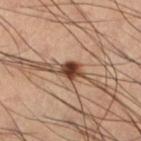biopsy status — imaged on a skin check; not biopsied
location — the left lower leg
lighting — cross-polarized illumination
automated metrics — an eccentricity of roughly 0.45 and two-axis asymmetry of about 0.2; a mean CIELAB color near L≈38 a*≈22 b*≈29, about 21 CIELAB-L* units darker than the surrounding skin, and a normalized lesion–skin contrast near 15.5; a border-irregularity index near 1.5/10, a within-lesion color-variation index near 2.5/10, and a peripheral color-asymmetry measure near 1
lesion diameter — ~2 mm (longest diameter)
subject — male, in their mid- to late 60s
acquisition — 15 mm crop, total-body photography